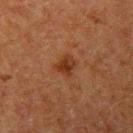Recorded during total-body skin imaging; not selected for excision or biopsy.